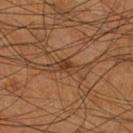Impression:
The lesion was photographed on a routine skin check and not biopsied; there is no pathology result.
Clinical summary:
A roughly 15 mm field-of-view crop from a total-body skin photograph. The lesion is located on the right lower leg. Longest diameter approximately 3.5 mm. Captured under cross-polarized illumination. A male subject, aged 53 to 57.